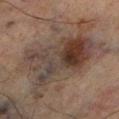notes — catalogued during a skin exam; not biopsied | subject — male, aged 63 to 67 | TBP lesion metrics — an outline eccentricity of about 0.85 (0 = round, 1 = elongated) and two-axis asymmetry of about 0.35; a border-irregularity index near 7.5/10, internal color variation of about 8 on a 0–10 scale, and peripheral color asymmetry of about 2.5; an automated nevus-likeness rating near 60 out of 100 and lesion-presence confidence of about 95/100 | imaging modality — ~15 mm tile from a whole-body skin photo | diameter — about 11.5 mm | location — the right lower leg.